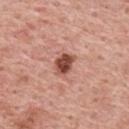biopsy_status: not biopsied; imaged during a skin examination
image:
  source: total-body photography crop
  field_of_view_mm: 15
lighting: white-light
patient:
  sex: male
  age_approx: 60
automated_metrics:
  area_mm2_approx: 5.5
  eccentricity: 0.7
  shape_asymmetry: 0.2
  cielab_L: 49
  cielab_a: 26
  cielab_b: 28
  vs_skin_darker_L: 16.0
  peripheral_color_asymmetry: 1.5
  nevus_likeness_0_100: 90
  lesion_detection_confidence_0_100: 100
lesion_size:
  long_diameter_mm_approx: 3.0
site: upper back